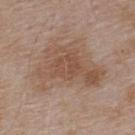The lesion was tiled from a total-body skin photograph and was not biopsied. A male subject aged around 55. From the upper back. Automated image analysis of the tile measured a lesion area of about 34 mm², an eccentricity of roughly 0.7, and a shape-asymmetry score of about 0.45 (0 = symmetric). It also reported internal color variation of about 4.5 on a 0–10 scale. The analysis additionally found a nevus-likeness score of about 0/100 and lesion-presence confidence of about 100/100. A 15 mm close-up tile from a total-body photography series done for melanoma screening. About 8.5 mm across.